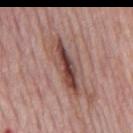notes — catalogued during a skin exam; not biopsied
anatomic site — the mid back
automated lesion analysis — a shape eccentricity near 0.95 and two-axis asymmetry of about 0.25; a mean CIELAB color near L≈47 a*≈21 b*≈23, about 12 CIELAB-L* units darker than the surrounding skin, and a lesion-to-skin contrast of about 9 (normalized; higher = more distinct)
acquisition — ~15 mm crop, total-body skin-cancer survey
patient — male, aged around 75